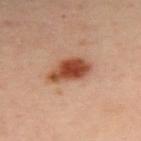This lesion was catalogued during total-body skin photography and was not selected for biopsy.
A 15 mm close-up tile from a total-body photography series done for melanoma screening.
A female subject aged approximately 40.
The lesion is located on the upper back.
Imaged with cross-polarized lighting.
Approximately 5 mm at its widest.
An algorithmic analysis of the crop reported an area of roughly 11 mm², an outline eccentricity of about 0.85 (0 = round, 1 = elongated), and two-axis asymmetry of about 0.25. It also reported a mean CIELAB color near L≈48 a*≈26 b*≈33, a lesion–skin lightness drop of about 16, and a normalized lesion–skin contrast near 11.5.